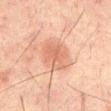<lesion>
<biopsy_status>not biopsied; imaged during a skin examination</biopsy_status>
<site>mid back</site>
<lesion_size>
  <long_diameter_mm_approx>4.0</long_diameter_mm_approx>
</lesion_size>
<patient>
  <sex>male</sex>
  <age_approx>65</age_approx>
</patient>
<lighting>cross-polarized</lighting>
<image>
  <source>total-body photography crop</source>
  <field_of_view_mm>15</field_of_view_mm>
</image>
</lesion>